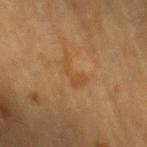workup: imaged on a skin check; not biopsied
automated metrics: an area of roughly 4.5 mm², a shape eccentricity near 0.9, and two-axis asymmetry of about 0.65; an average lesion color of about L≈42 a*≈18 b*≈35 (CIELAB) and a lesion-to-skin contrast of about 5 (normalized; higher = more distinct)
illumination: cross-polarized
subject: male, aged approximately 85 or older
body site: the left upper arm
image: total-body-photography crop, ~15 mm field of view
lesion diameter: ~3.5 mm (longest diameter)